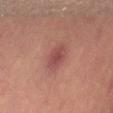Notes:
– notes — imaged on a skin check; not biopsied
– acquisition — 15 mm crop, total-body photography
– body site — the right thigh
– subject — female, in their mid- to late 40s
– lighting — cross-polarized
– lesion size — about 3.5 mm